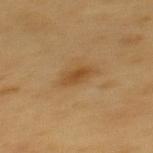Impression:
The lesion was tiled from a total-body skin photograph and was not biopsied.
Clinical summary:
A male subject approximately 60 years of age. This image is a 15 mm lesion crop taken from a total-body photograph. On the back. This is a cross-polarized tile. Approximately 3 mm at its widest.